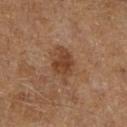This lesion was catalogued during total-body skin photography and was not selected for biopsy.
A 15 mm crop from a total-body photograph taken for skin-cancer surveillance.
A male patient aged 58–62.
Captured under cross-polarized illumination.
The lesion is on the right lower leg.
Longest diameter approximately 4.5 mm.